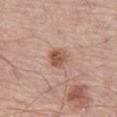Recorded during total-body skin imaging; not selected for excision or biopsy.